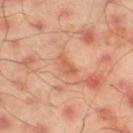Part of a total-body skin-imaging series; this lesion was reviewed on a skin check and was not flagged for biopsy. A male subject, aged 43 to 47. About 3 mm across. The total-body-photography lesion software estimated a footprint of about 3.5 mm² and two-axis asymmetry of about 0.35. And it measured a border-irregularity rating of about 3.5/10, a within-lesion color-variation index near 2/10, and radial color variation of about 0.5. And it measured a lesion-detection confidence of about 100/100. A 15 mm close-up extracted from a 3D total-body photography capture. The lesion is located on the left thigh.